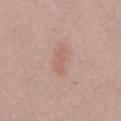The tile uses white-light illumination.
On the chest.
A male patient, approximately 40 years of age.
Cropped from a total-body skin-imaging series; the visible field is about 15 mm.
Longest diameter approximately 3.5 mm.
Automated image analysis of the tile measured an area of roughly 4 mm², an outline eccentricity of about 0.9 (0 = round, 1 = elongated), and two-axis asymmetry of about 0.3. It also reported a border-irregularity rating of about 3.5/10, a within-lesion color-variation index near 1/10, and radial color variation of about 0. It also reported a classifier nevus-likeness of about 0/100 and a detector confidence of about 100 out of 100 that the crop contains a lesion.